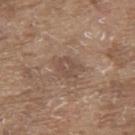{
  "biopsy_status": "not biopsied; imaged during a skin examination",
  "patient": {
    "sex": "male",
    "age_approx": 80
  },
  "lighting": "white-light",
  "image": {
    "source": "total-body photography crop",
    "field_of_view_mm": 15
  },
  "site": "upper back"
}